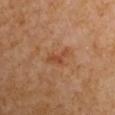Clinical impression:
This lesion was catalogued during total-body skin photography and was not selected for biopsy.
Clinical summary:
The recorded lesion diameter is about 3 mm. Automated tile analysis of the lesion measured a mean CIELAB color near L≈46 a*≈24 b*≈34, a lesion–skin lightness drop of about 7, and a lesion-to-skin contrast of about 6 (normalized; higher = more distinct). And it measured border irregularity of about 4.5 on a 0–10 scale, a color-variation rating of about 2/10, and peripheral color asymmetry of about 0.5. The analysis additionally found an automated nevus-likeness rating near 0 out of 100 and a lesion-detection confidence of about 100/100. The patient is a male approximately 55 years of age. A region of skin cropped from a whole-body photographic capture, roughly 15 mm wide. The tile uses cross-polarized illumination. The lesion is located on the right upper arm.